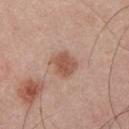This lesion was catalogued during total-body skin photography and was not selected for biopsy. This image is a 15 mm lesion crop taken from a total-body photograph. Automated tile analysis of the lesion measured border irregularity of about 2.5 on a 0–10 scale, a within-lesion color-variation index near 2.5/10, and a peripheral color-asymmetry measure near 0.5. The analysis additionally found lesion-presence confidence of about 100/100. A male subject approximately 25 years of age. From the chest. Measured at roughly 3 mm in maximum diameter. Captured under white-light illumination.